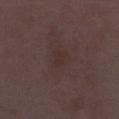This lesion was catalogued during total-body skin photography and was not selected for biopsy.